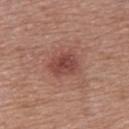Approximately 3.5 mm at its widest. The patient is a female aged 38 to 42. Located on the back. Imaged with white-light lighting. A 15 mm crop from a total-body photograph taken for skin-cancer surveillance.